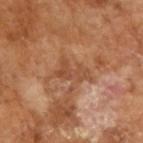Assessment: No biopsy was performed on this lesion — it was imaged during a full skin examination and was not determined to be concerning. Image and clinical context: The recorded lesion diameter is about 3.5 mm. Imaged with cross-polarized lighting. A close-up tile cropped from a whole-body skin photograph, about 15 mm across. A male patient, in their mid- to late 60s.